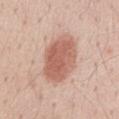{"biopsy_status": "not biopsied; imaged during a skin examination", "image": {"source": "total-body photography crop", "field_of_view_mm": 15}, "lighting": "white-light", "patient": {"sex": "male", "age_approx": 60}, "site": "abdomen"}